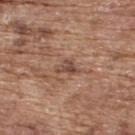| key | value |
|---|---|
| workup | no biopsy performed (imaged during a skin exam) |
| tile lighting | white-light |
| subject | male, aged around 75 |
| lesion size | ~3 mm (longest diameter) |
| imaging modality | 15 mm crop, total-body photography |
| site | the upper back |